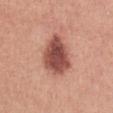Part of a total-body skin-imaging series; this lesion was reviewed on a skin check and was not flagged for biopsy. The lesion is located on the front of the torso. A female patient in their mid-20s. This is a white-light tile. A 15 mm close-up extracted from a 3D total-body photography capture. Automated image analysis of the tile measured a mean CIELAB color near L≈51 a*≈26 b*≈27, a lesion–skin lightness drop of about 16, and a lesion-to-skin contrast of about 11 (normalized; higher = more distinct). And it measured border irregularity of about 2 on a 0–10 scale and a peripheral color-asymmetry measure near 1.5. The software also gave an automated nevus-likeness rating near 60 out of 100.